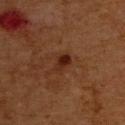Q: Was this lesion biopsied?
A: catalogued during a skin exam; not biopsied
Q: What is the lesion's diameter?
A: about 2.5 mm
Q: Patient demographics?
A: male, approximately 65 years of age
Q: What is the anatomic site?
A: the back
Q: What is the imaging modality?
A: 15 mm crop, total-body photography